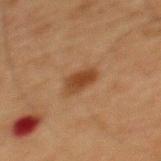Recorded during total-body skin imaging; not selected for excision or biopsy.
The subject is a male roughly 65 years of age.
Cropped from a total-body skin-imaging series; the visible field is about 15 mm.
The recorded lesion diameter is about 3.5 mm.
Captured under cross-polarized illumination.
On the mid back.